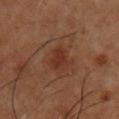The lesion was tiled from a total-body skin photograph and was not biopsied.
Automated tile analysis of the lesion measured about 7 CIELAB-L* units darker than the surrounding skin. The software also gave a border-irregularity rating of about 3/10, a color-variation rating of about 2.5/10, and radial color variation of about 1.
On the chest.
Measured at roughly 3 mm in maximum diameter.
A 15 mm crop from a total-body photograph taken for skin-cancer surveillance.
The tile uses cross-polarized illumination.
The patient is a male aged 53 to 57.